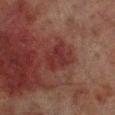Findings:
- biopsy status · no biopsy performed (imaged during a skin exam)
- image · total-body-photography crop, ~15 mm field of view
- site · the right lower leg
- patient · male, about 70 years old